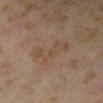Measured at roughly 4.5 mm in maximum diameter. A female subject, in their 40s. Captured under cross-polarized illumination. A 15 mm close-up extracted from a 3D total-body photography capture. Located on the right lower leg.